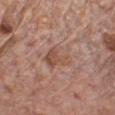{"biopsy_status": "not biopsied; imaged during a skin examination", "site": "chest", "patient": {"sex": "male", "age_approx": 80}, "image": {"source": "total-body photography crop", "field_of_view_mm": 15}, "automated_metrics": {"area_mm2_approx": 8.0, "eccentricity": 0.65, "border_irregularity_0_10": 2.5, "color_variation_0_10": 6.0}, "lighting": "white-light", "lesion_size": {"long_diameter_mm_approx": 3.5}}